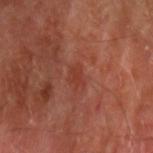<tbp_lesion>
  <biopsy_status>not biopsied; imaged during a skin examination</biopsy_status>
  <patient>
    <sex>male</sex>
    <age_approx>70</age_approx>
  </patient>
  <image>
    <source>total-body photography crop</source>
    <field_of_view_mm>15</field_of_view_mm>
  </image>
  <site>left upper arm</site>
</tbp_lesion>